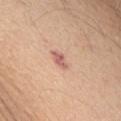This lesion was catalogued during total-body skin photography and was not selected for biopsy. This image is a 15 mm lesion crop taken from a total-body photograph. Approximately 2.5 mm at its widest. This is a white-light tile. From the abdomen. A male subject roughly 70 years of age. The lesion-visualizer software estimated a border-irregularity index near 3.5/10, internal color variation of about 1.5 on a 0–10 scale, and radial color variation of about 0.5. The analysis additionally found a classifier nevus-likeness of about 0/100.